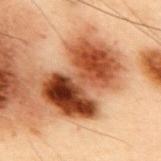| key | value |
|---|---|
| workup | imaged on a skin check; not biopsied |
| patient | male, aged approximately 55 |
| lesion size | about 7.5 mm |
| illumination | cross-polarized |
| imaging modality | total-body-photography crop, ~15 mm field of view |
| body site | the mid back |